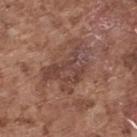Recorded during total-body skin imaging; not selected for excision or biopsy. Measured at roughly 6.5 mm in maximum diameter. A close-up tile cropped from a whole-body skin photograph, about 15 mm across. The lesion-visualizer software estimated a lesion area of about 17 mm², a shape eccentricity near 0.75, and a shape-asymmetry score of about 0.5 (0 = symmetric). The analysis additionally found a classifier nevus-likeness of about 0/100 and lesion-presence confidence of about 75/100. From the upper back. A male subject, aged 73 to 77.